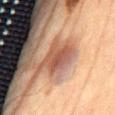The lesion was photographed on a routine skin check and not biopsied; there is no pathology result.
An algorithmic analysis of the crop reported an average lesion color of about L≈44 a*≈20 b*≈26 (CIELAB), roughly 9 lightness units darker than nearby skin, and a normalized border contrast of about 7. It also reported border irregularity of about 3.5 on a 0–10 scale, internal color variation of about 3.5 on a 0–10 scale, and a peripheral color-asymmetry measure near 1. It also reported a nevus-likeness score of about 10/100.
Located on the abdomen.
A region of skin cropped from a whole-body photographic capture, roughly 15 mm wide.
A male patient aged 68–72.